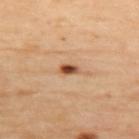notes = catalogued during a skin exam; not biopsied | diameter = about 2.5 mm | subject = female, aged around 45 | tile lighting = cross-polarized illumination | site = the upper back | imaging modality = total-body-photography crop, ~15 mm field of view.